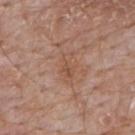biopsy status=imaged on a skin check; not biopsied
lesion diameter=~3.5 mm (longest diameter)
automated metrics=an average lesion color of about L≈52 a*≈21 b*≈30 (CIELAB), about 6 CIELAB-L* units darker than the surrounding skin, and a normalized border contrast of about 5.5; a border-irregularity index near 4.5/10, a within-lesion color-variation index near 2.5/10, and radial color variation of about 1; a nevus-likeness score of about 0/100 and a detector confidence of about 100 out of 100 that the crop contains a lesion
patient=male, in their 60s
body site=the mid back
imaging modality=15 mm crop, total-body photography